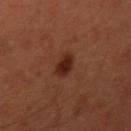Clinical impression: Imaged during a routine full-body skin examination; the lesion was not biopsied and no histopathology is available. Image and clinical context: Cropped from a total-body skin-imaging series; the visible field is about 15 mm. About 2.5 mm across. Located on the arm. A male subject aged 33–37. Imaged with cross-polarized lighting. Automated tile analysis of the lesion measured border irregularity of about 2 on a 0–10 scale, a within-lesion color-variation index near 1.5/10, and radial color variation of about 0.5. The software also gave lesion-presence confidence of about 100/100.